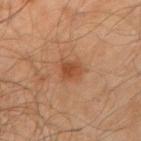Findings:
- follow-up — no biopsy performed (imaged during a skin exam)
- lighting — cross-polarized
- imaging modality — 15 mm crop, total-body photography
- lesion diameter — about 3.5 mm
- patient — male, aged 63–67
- anatomic site — the arm